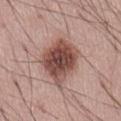Impression:
Recorded during total-body skin imaging; not selected for excision or biopsy.
Image and clinical context:
A region of skin cropped from a whole-body photographic capture, roughly 15 mm wide. Captured under white-light illumination. On the abdomen. Automated image analysis of the tile measured an average lesion color of about L≈49 a*≈21 b*≈24 (CIELAB). It also reported a border-irregularity rating of about 2.5/10, a color-variation rating of about 6.5/10, and peripheral color asymmetry of about 2. The analysis additionally found a nevus-likeness score of about 90/100 and a detector confidence of about 100 out of 100 that the crop contains a lesion. A male patient aged around 55. The lesion's longest dimension is about 6 mm.